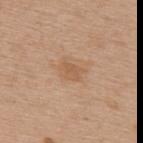Impression:
Recorded during total-body skin imaging; not selected for excision or biopsy.
Image and clinical context:
The recorded lesion diameter is about 3 mm. A roughly 15 mm field-of-view crop from a total-body skin photograph. A female subject, in their mid-60s. The lesion is on the upper back. Automated image analysis of the tile measured an area of roughly 4.5 mm², an eccentricity of roughly 0.75, and a symmetry-axis asymmetry near 0.25. It also reported a classifier nevus-likeness of about 0/100 and lesion-presence confidence of about 100/100.